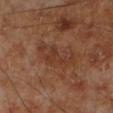Recorded during total-body skin imaging; not selected for excision or biopsy. A male patient, roughly 70 years of age. A roughly 15 mm field-of-view crop from a total-body skin photograph. Located on the left lower leg.